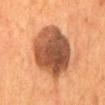Part of a total-body skin-imaging series; this lesion was reviewed on a skin check and was not flagged for biopsy.
The lesion is on the mid back.
The patient is a male approximately 55 years of age.
A region of skin cropped from a whole-body photographic capture, roughly 15 mm wide.
The recorded lesion diameter is about 7.5 mm.